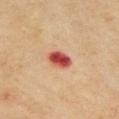Assessment:
The lesion was photographed on a routine skin check and not biopsied; there is no pathology result.
Image and clinical context:
The tile uses cross-polarized illumination. Measured at roughly 3 mm in maximum diameter. The lesion is on the chest. A male subject, aged 68–72. A 15 mm close-up extracted from a 3D total-body photography capture.